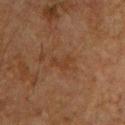Clinical impression:
The lesion was tiled from a total-body skin photograph and was not biopsied.
Image and clinical context:
The total-body-photography lesion software estimated a footprint of about 3 mm², an outline eccentricity of about 0.85 (0 = round, 1 = elongated), and two-axis asymmetry of about 0.55. And it measured a mean CIELAB color near L≈30 a*≈17 b*≈27. The analysis additionally found an automated nevus-likeness rating near 0 out of 100 and a detector confidence of about 100 out of 100 that the crop contains a lesion. Imaged with cross-polarized lighting. Located on the front of the torso. A lesion tile, about 15 mm wide, cut from a 3D total-body photograph. A male patient aged around 65.